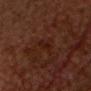Notes:
- biopsy status · imaged on a skin check; not biopsied
- acquisition · total-body-photography crop, ~15 mm field of view
- anatomic site · the head or neck
- patient · male, aged 63–67